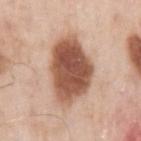Assessment:
Imaged during a routine full-body skin examination; the lesion was not biopsied and no histopathology is available.
Acquisition and patient details:
A male patient, in their mid- to late 50s. This is a white-light tile. The total-body-photography lesion software estimated an average lesion color of about L≈54 a*≈22 b*≈30 (CIELAB) and a normalized border contrast of about 12. And it measured border irregularity of about 2.5 on a 0–10 scale. Located on the left upper arm. This image is a 15 mm lesion crop taken from a total-body photograph. About 7.5 mm across.